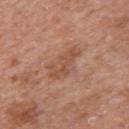follow-up: imaged on a skin check; not biopsied
imaging modality: ~15 mm tile from a whole-body skin photo
illumination: white-light illumination
subject: male, aged 63–67
body site: the left upper arm
size: about 4.5 mm
TBP lesion metrics: a lesion area of about 7.5 mm², an eccentricity of roughly 0.9, and two-axis asymmetry of about 0.4; a mean CIELAB color near L≈51 a*≈22 b*≈30, roughly 8 lightness units darker than nearby skin, and a lesion-to-skin contrast of about 6 (normalized; higher = more distinct); an automated nevus-likeness rating near 0 out of 100 and a lesion-detection confidence of about 100/100